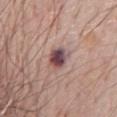Cropped from a whole-body photographic skin survey; the tile spans about 15 mm.
On the chest.
A male subject, approximately 70 years of age.
Captured under white-light illumination.
About 3 mm across.